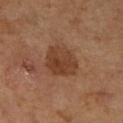Q: Is there a histopathology result?
A: no biopsy performed (imaged during a skin exam)
Q: Lesion size?
A: about 4 mm
Q: How was the tile lit?
A: cross-polarized illumination
Q: How was this image acquired?
A: ~15 mm tile from a whole-body skin photo
Q: Where on the body is the lesion?
A: the right lower leg
Q: Patient demographics?
A: female, aged approximately 65